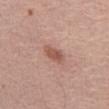The lesion was photographed on a routine skin check and not biopsied; there is no pathology result. The lesion is located on the abdomen. A female subject in their mid- to late 60s. A 15 mm close-up tile from a total-body photography series done for melanoma screening. The lesion's longest dimension is about 3 mm.